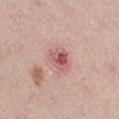workup: imaged on a skin check; not biopsied
image: ~15 mm tile from a whole-body skin photo
automated lesion analysis: an average lesion color of about L≈57 a*≈27 b*≈22 (CIELAB) and a normalized lesion–skin contrast near 8; a border-irregularity index near 2/10 and a within-lesion color-variation index near 7/10
patient: female, about 40 years old
lesion diameter: ≈3 mm
tile lighting: white-light
anatomic site: the leg